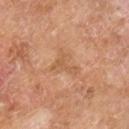<record>
<biopsy_status>not biopsied; imaged during a skin examination</biopsy_status>
<image>
  <source>total-body photography crop</source>
  <field_of_view_mm>15</field_of_view_mm>
</image>
<site>right lower leg</site>
<patient>
  <sex>male</sex>
  <age_approx>65</age_approx>
</patient>
<automated_metrics>
  <cielab_L>58</cielab_L>
  <cielab_a>22</cielab_a>
  <cielab_b>36</cielab_b>
  <vs_skin_darker_L>6.0</vs_skin_darker_L>
  <vs_skin_contrast_norm>4.5</vs_skin_contrast_norm>
</automated_metrics>
<lighting>cross-polarized</lighting>
<lesion_size>
  <long_diameter_mm_approx>3.5</long_diameter_mm_approx>
</lesion_size>
</record>